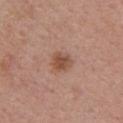| key | value |
|---|---|
| notes | total-body-photography surveillance lesion; no biopsy |
| patient | female, aged 48 to 52 |
| image | ~15 mm tile from a whole-body skin photo |
| illumination | white-light |
| site | the left upper arm |